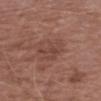The tile uses white-light illumination. An algorithmic analysis of the crop reported a footprint of about 7.5 mm². The software also gave roughly 6 lightness units darker than nearby skin and a normalized lesion–skin contrast near 5. The software also gave a color-variation rating of about 3/10 and radial color variation of about 1. The subject is a male in their mid- to late 60s. A close-up tile cropped from a whole-body skin photograph, about 15 mm across. From the right upper arm. The lesion's longest dimension is about 3.5 mm.